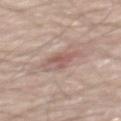Assessment: No biopsy was performed on this lesion — it was imaged during a full skin examination and was not determined to be concerning.